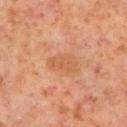This lesion was catalogued during total-body skin photography and was not selected for biopsy. Located on the left lower leg. A male patient aged 58–62. The tile uses cross-polarized illumination. About 4 mm across. A close-up tile cropped from a whole-body skin photograph, about 15 mm across.